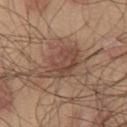biopsy status = catalogued during a skin exam; not biopsied
automated lesion analysis = an area of roughly 12 mm², a shape eccentricity near 0.8, and two-axis asymmetry of about 0.25
image = ~15 mm tile from a whole-body skin photo
lesion diameter = ≈5.5 mm
lighting = white-light
patient = male, aged around 45
anatomic site = the chest